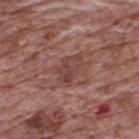Q: Was a biopsy performed?
A: no biopsy performed (imaged during a skin exam)
Q: What is the imaging modality?
A: 15 mm crop, total-body photography
Q: What is the anatomic site?
A: the upper back
Q: Patient demographics?
A: male, aged 68 to 72
Q: Lesion size?
A: about 4 mm
Q: What lighting was used for the tile?
A: white-light